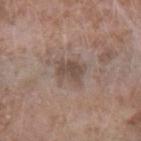This is a white-light tile. A female patient, approximately 75 years of age. This image is a 15 mm lesion crop taken from a total-body photograph. Measured at roughly 3 mm in maximum diameter. On the left forearm. Automated image analysis of the tile measured a lesion area of about 6 mm², an eccentricity of roughly 0.5, and a symmetry-axis asymmetry near 0.2. The software also gave a lesion color around L≈48 a*≈14 b*≈23 in CIELAB and a normalized border contrast of about 6.5. The software also gave border irregularity of about 2.5 on a 0–10 scale and a peripheral color-asymmetry measure near 0.5. The analysis additionally found an automated nevus-likeness rating near 0 out of 100 and lesion-presence confidence of about 100/100.